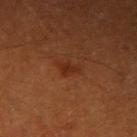Case summary:
– biopsy status · no biopsy performed (imaged during a skin exam)
– location · the right upper arm
– lesion diameter · about 2.5 mm
– image source · ~15 mm crop, total-body skin-cancer survey
– illumination · cross-polarized illumination
– patient · male, in their 60s